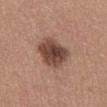Q: Was a biopsy performed?
A: catalogued during a skin exam; not biopsied
Q: Patient demographics?
A: male, in their mid-20s
Q: How was this image acquired?
A: total-body-photography crop, ~15 mm field of view
Q: How was the tile lit?
A: white-light
Q: Where on the body is the lesion?
A: the abdomen
Q: Automated lesion metrics?
A: a shape eccentricity near 0.6; an average lesion color of about L≈45 a*≈19 b*≈25 (CIELAB), roughly 15 lightness units darker than nearby skin, and a lesion-to-skin contrast of about 11 (normalized; higher = more distinct); a border-irregularity index near 2/10, internal color variation of about 5 on a 0–10 scale, and radial color variation of about 1.5